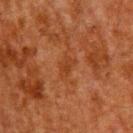The lesion was photographed on a routine skin check and not biopsied; there is no pathology result.
About 2.5 mm across.
The subject is a male aged 58–62.
A lesion tile, about 15 mm wide, cut from a 3D total-body photograph.
On the back.
An algorithmic analysis of the crop reported an area of roughly 3.5 mm², an outline eccentricity of about 0.8 (0 = round, 1 = elongated), and two-axis asymmetry of about 0.3. And it measured a mean CIELAB color near L≈31 a*≈22 b*≈31 and a lesion-to-skin contrast of about 5.5 (normalized; higher = more distinct).
Imaged with cross-polarized lighting.